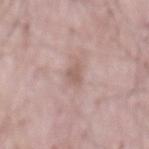No biopsy was performed on this lesion — it was imaged during a full skin examination and was not determined to be concerning. The recorded lesion diameter is about 3 mm. The tile uses white-light illumination. A close-up tile cropped from a whole-body skin photograph, about 15 mm across. On the abdomen. A male subject in their mid- to late 60s. An algorithmic analysis of the crop reported a footprint of about 3.5 mm², an eccentricity of roughly 0.9, and two-axis asymmetry of about 0.3. The software also gave a border-irregularity index near 3.5/10 and internal color variation of about 1 on a 0–10 scale. The software also gave an automated nevus-likeness rating near 0 out of 100 and lesion-presence confidence of about 95/100.